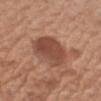Assessment:
The lesion was tiled from a total-body skin photograph and was not biopsied.
Context:
From the arm. A female subject, roughly 75 years of age. A 15 mm crop from a total-body photograph taken for skin-cancer surveillance.